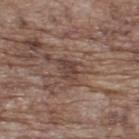Case summary:
- workup: catalogued during a skin exam; not biopsied
- body site: the back
- automated lesion analysis: a lesion area of about 4 mm², an outline eccentricity of about 0.65 (0 = round, 1 = elongated), and a shape-asymmetry score of about 0.35 (0 = symmetric); a border-irregularity index near 3.5/10 and peripheral color asymmetry of about 1
- patient: male, aged approximately 70
- lighting: white-light illumination
- image: ~15 mm crop, total-body skin-cancer survey